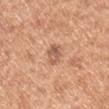Captured during whole-body skin photography for melanoma surveillance; the lesion was not biopsied. Located on the right upper arm. A region of skin cropped from a whole-body photographic capture, roughly 15 mm wide. A male subject, in their mid- to late 50s.